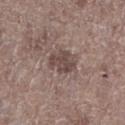Captured during whole-body skin photography for melanoma surveillance; the lesion was not biopsied.
Cropped from a whole-body photographic skin survey; the tile spans about 15 mm.
Imaged with white-light lighting.
The lesion-visualizer software estimated a shape-asymmetry score of about 0.4 (0 = symmetric). The software also gave a border-irregularity index near 4.5/10, a within-lesion color-variation index near 2/10, and peripheral color asymmetry of about 1.
The recorded lesion diameter is about 3 mm.
From the right lower leg.
A male subject, aged 68–72.